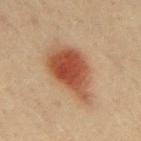- biopsy status — no biopsy performed (imaged during a skin exam)